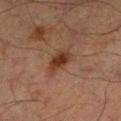<tbp_lesion>
  <image>
    <source>total-body photography crop</source>
    <field_of_view_mm>15</field_of_view_mm>
  </image>
  <site>right lower leg</site>
  <patient>
    <sex>male</sex>
    <age_approx>65</age_approx>
  </patient>
</tbp_lesion>